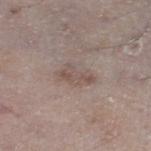Q: What kind of image is this?
A: 15 mm crop, total-body photography
Q: What are the patient's age and sex?
A: male, roughly 65 years of age
Q: What is the anatomic site?
A: the right thigh